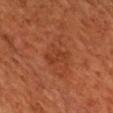<tbp_lesion>
  <biopsy_status>not biopsied; imaged during a skin examination</biopsy_status>
  <site>chest</site>
  <image>
    <source>total-body photography crop</source>
    <field_of_view_mm>15</field_of_view_mm>
  </image>
  <patient>
    <sex>male</sex>
    <age_approx>65</age_approx>
  </patient>
  <lighting>cross-polarized</lighting>
</tbp_lesion>